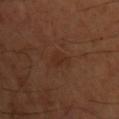<tbp_lesion>
<automated_metrics>
  <nevus_likeness_0_100>0</nevus_likeness_0_100>
  <lesion_detection_confidence_0_100>100</lesion_detection_confidence_0_100>
</automated_metrics>
<image>
  <source>total-body photography crop</source>
  <field_of_view_mm>15</field_of_view_mm>
</image>
<site>chest</site>
<lesion_size>
  <long_diameter_mm_approx>3.0</long_diameter_mm_approx>
</lesion_size>
<lighting>cross-polarized</lighting>
<patient>
  <sex>male</sex>
  <age_approx>55</age_approx>
</patient>
</tbp_lesion>